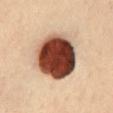Impression: Captured during whole-body skin photography for melanoma surveillance; the lesion was not biopsied. Clinical summary: A female subject roughly 35 years of age. A 15 mm crop from a total-body photograph taken for skin-cancer surveillance. Located on the chest.